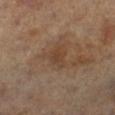biopsy status — total-body-photography surveillance lesion; no biopsy
acquisition — total-body-photography crop, ~15 mm field of view
body site — the left leg
image-analysis metrics — a lesion color around L≈39 a*≈17 b*≈28 in CIELAB, a lesion–skin lightness drop of about 6, and a lesion-to-skin contrast of about 5 (normalized; higher = more distinct)
subject — female, approximately 65 years of age